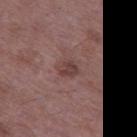Notes:
– workup — imaged on a skin check; not biopsied
– subject — male, roughly 50 years of age
– site — the left thigh
– image — total-body-photography crop, ~15 mm field of view
– lesion diameter — ≈2.5 mm